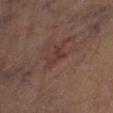biopsy status = total-body-photography surveillance lesion; no biopsy
size = about 3 mm
patient = male, aged 83 or older
location = the right lower leg
image source = total-body-photography crop, ~15 mm field of view
tile lighting = cross-polarized